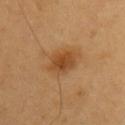Q: Was a biopsy performed?
A: total-body-photography surveillance lesion; no biopsy
Q: What lighting was used for the tile?
A: cross-polarized
Q: What is the imaging modality?
A: 15 mm crop, total-body photography
Q: Who is the patient?
A: male, in their 60s
Q: Automated lesion metrics?
A: a footprint of about 8.5 mm² and two-axis asymmetry of about 0.15; a border-irregularity index near 1.5/10, a within-lesion color-variation index near 4/10, and peripheral color asymmetry of about 1
Q: What is the lesion's diameter?
A: ≈4 mm
Q: What is the anatomic site?
A: the front of the torso